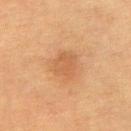Assessment: Imaged during a routine full-body skin examination; the lesion was not biopsied and no histopathology is available. Acquisition and patient details: On the chest. A lesion tile, about 15 mm wide, cut from a 3D total-body photograph. The lesion's longest dimension is about 3 mm. A female patient, in their mid- to late 50s. The tile uses cross-polarized illumination.